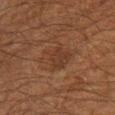– site: the leg
– imaging modality: 15 mm crop, total-body photography
– patient: male, approximately 60 years of age